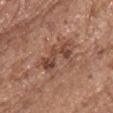Part of a total-body skin-imaging series; this lesion was reviewed on a skin check and was not flagged for biopsy. A 15 mm close-up tile from a total-body photography series done for melanoma screening. The lesion is on the chest. The total-body-photography lesion software estimated a footprint of about 7.5 mm² and a shape-asymmetry score of about 0.5 (0 = symmetric). It also reported a within-lesion color-variation index near 3/10 and radial color variation of about 1. The subject is a female in their mid- to late 60s.